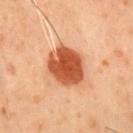The lesion was photographed on a routine skin check and not biopsied; there is no pathology result.
The tile uses cross-polarized illumination.
A male patient in their mid- to late 50s.
A roughly 15 mm field-of-view crop from a total-body skin photograph.
From the chest.
The total-body-photography lesion software estimated an eccentricity of roughly 0.5 and a symmetry-axis asymmetry near 0.1. The analysis additionally found a mean CIELAB color near L≈44 a*≈26 b*≈34, a lesion–skin lightness drop of about 15, and a lesion-to-skin contrast of about 11.5 (normalized; higher = more distinct). And it measured a nevus-likeness score of about 100/100.
About 5 mm across.